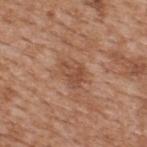Impression:
No biopsy was performed on this lesion — it was imaged during a full skin examination and was not determined to be concerning.
Acquisition and patient details:
Cropped from a total-body skin-imaging series; the visible field is about 15 mm. Measured at roughly 3.5 mm in maximum diameter. Captured under white-light illumination. The lesion is located on the upper back. The subject is a male approximately 65 years of age.